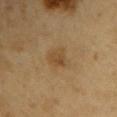The lesion was photographed on a routine skin check and not biopsied; there is no pathology result. About 3 mm across. Imaged with cross-polarized lighting. A close-up tile cropped from a whole-body skin photograph, about 15 mm across. The patient is a male about 60 years old. From the arm.